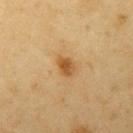Notes:
* lighting: cross-polarized
* diameter: ≈2.5 mm
* acquisition: ~15 mm crop, total-body skin-cancer survey
* anatomic site: the left upper arm
* patient: male, aged approximately 60